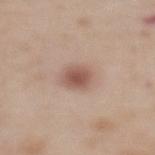  image:
    source: total-body photography crop
    field_of_view_mm: 15
  patient:
    sex: female
    age_approx: 65
  site: mid back
  lighting: white-light
  lesion_size:
    long_diameter_mm_approx: 3.0